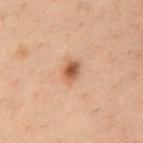Part of a total-body skin-imaging series; this lesion was reviewed on a skin check and was not flagged for biopsy. The lesion-visualizer software estimated a lesion area of about 4.5 mm², a shape eccentricity near 0.75, and a symmetry-axis asymmetry near 0.2. It also reported a mean CIELAB color near L≈45 a*≈19 b*≈28, about 11 CIELAB-L* units darker than the surrounding skin, and a normalized lesion–skin contrast near 8.5. The software also gave border irregularity of about 2 on a 0–10 scale and radial color variation of about 1.5. It also reported a classifier nevus-likeness of about 90/100 and a lesion-detection confidence of about 100/100. The recorded lesion diameter is about 3 mm. A male patient roughly 40 years of age. This is a cross-polarized tile. From the chest. A 15 mm crop from a total-body photograph taken for skin-cancer surveillance.